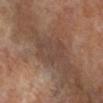{"site": "left lower leg", "automated_metrics": {"shape_asymmetry": 0.35}, "image": {"source": "total-body photography crop", "field_of_view_mm": 15}, "patient": {"sex": "female", "age_approx": 75}, "lesion_size": {"long_diameter_mm_approx": 4.5}, "lighting": "cross-polarized"}